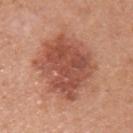Part of a total-body skin-imaging series; this lesion was reviewed on a skin check and was not flagged for biopsy. The recorded lesion diameter is about 7 mm. The lesion is on the left upper arm. The tile uses white-light illumination. This image is a 15 mm lesion crop taken from a total-body photograph. The subject is a female approximately 50 years of age.